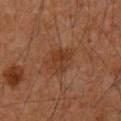The lesion was tiled from a total-body skin photograph and was not biopsied.
A 15 mm close-up tile from a total-body photography series done for melanoma screening.
Automated image analysis of the tile measured a mean CIELAB color near L≈33 a*≈21 b*≈29 and roughly 6 lightness units darker than nearby skin. And it measured border irregularity of about 2.5 on a 0–10 scale and internal color variation of about 3.5 on a 0–10 scale.
The patient is a male in their 60s.
On the mid back.
Imaged with cross-polarized lighting.
Longest diameter approximately 3.5 mm.